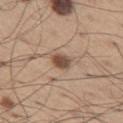Assessment: Part of a total-body skin-imaging series; this lesion was reviewed on a skin check and was not flagged for biopsy. Background: A lesion tile, about 15 mm wide, cut from a 3D total-body photograph. Imaged with white-light lighting. From the left thigh. An algorithmic analysis of the crop reported an area of roughly 4.5 mm², a shape eccentricity near 0.7, and a symmetry-axis asymmetry near 0.25. It also reported about 14 CIELAB-L* units darker than the surrounding skin and a normalized border contrast of about 9.5. It also reported a within-lesion color-variation index near 3/10 and radial color variation of about 1. A male subject, aged 63 to 67. Longest diameter approximately 2.5 mm.